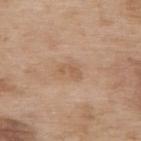* follow-up — no biopsy performed (imaged during a skin exam)
* diameter — about 2.5 mm
* anatomic site — the upper back
* image — ~15 mm crop, total-body skin-cancer survey
* image-analysis metrics — a footprint of about 2.5 mm², an outline eccentricity of about 0.85 (0 = round, 1 = elongated), and a shape-asymmetry score of about 0.4 (0 = symmetric); a nevus-likeness score of about 0/100 and a detector confidence of about 100 out of 100 that the crop contains a lesion
* patient — female, aged approximately 75
* illumination — white-light